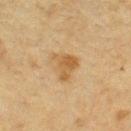A 15 mm close-up extracted from a 3D total-body photography capture. The lesion-visualizer software estimated a lesion area of about 6.5 mm², a shape eccentricity near 0.8, and a symmetry-axis asymmetry near 0.4. It also reported a lesion color around L≈48 a*≈16 b*≈36 in CIELAB, a lesion–skin lightness drop of about 8, and a normalized border contrast of about 7. And it measured a border-irregularity rating of about 4.5/10, a color-variation rating of about 3/10, and radial color variation of about 1. The software also gave an automated nevus-likeness rating near 15 out of 100. A female subject aged 53 to 57. The recorded lesion diameter is about 4 mm. From the left thigh.